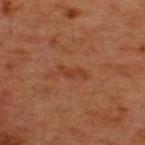Recorded during total-body skin imaging; not selected for excision or biopsy.
A roughly 15 mm field-of-view crop from a total-body skin photograph.
The subject is a male aged around 50.
Measured at roughly 3 mm in maximum diameter.
The tile uses cross-polarized illumination.
The lesion is on the upper back.